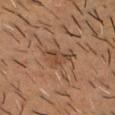notes=catalogued during a skin exam; not biopsied
subject=male, aged 58–62
site=the head or neck
size=~3.5 mm (longest diameter)
illumination=cross-polarized
automated metrics=an area of roughly 5 mm², a shape eccentricity near 0.75, and two-axis asymmetry of about 0.5; a mean CIELAB color near L≈36 a*≈16 b*≈26 and a lesion–skin lightness drop of about 6; a border-irregularity index near 5.5/10 and a peripheral color-asymmetry measure near 1; an automated nevus-likeness rating near 0 out of 100 and lesion-presence confidence of about 85/100
acquisition=~15 mm tile from a whole-body skin photo